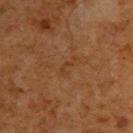Impression: Captured during whole-body skin photography for melanoma surveillance; the lesion was not biopsied. Image and clinical context: Measured at roughly 2.5 mm in maximum diameter. The subject is a male approximately 60 years of age. A 15 mm close-up extracted from a 3D total-body photography capture. Captured under cross-polarized illumination. The lesion is on the upper back.